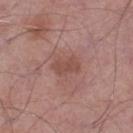follow-up = catalogued during a skin exam; not biopsied | image = ~15 mm crop, total-body skin-cancer survey | location = the leg | subject = male, approximately 55 years of age.